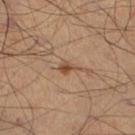Part of a total-body skin-imaging series; this lesion was reviewed on a skin check and was not flagged for biopsy. A lesion tile, about 15 mm wide, cut from a 3D total-body photograph. This is a cross-polarized tile. From the left leg. A male subject, in their 50s. The total-body-photography lesion software estimated a footprint of about 3 mm², an eccentricity of roughly 0.85, and a shape-asymmetry score of about 0.35 (0 = symmetric). Approximately 2.5 mm at its widest.